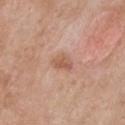Q: Was a biopsy performed?
A: total-body-photography surveillance lesion; no biopsy
Q: What is the anatomic site?
A: the chest
Q: How was the tile lit?
A: white-light
Q: Patient demographics?
A: female, in their 60s
Q: What is the lesion's diameter?
A: about 2.5 mm
Q: How was this image acquired?
A: ~15 mm tile from a whole-body skin photo